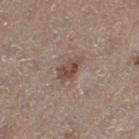{"biopsy_status": "not biopsied; imaged during a skin examination", "patient": {"sex": "female", "age_approx": 50}, "lighting": "white-light", "automated_metrics": {"area_mm2_approx": 5.5, "cielab_L": 47, "cielab_a": 16, "cielab_b": 24, "vs_skin_darker_L": 10.0, "vs_skin_contrast_norm": 8.0, "border_irregularity_0_10": 2.5, "color_variation_0_10": 3.5, "peripheral_color_asymmetry": 1.0, "lesion_detection_confidence_0_100": 100}, "lesion_size": {"long_diameter_mm_approx": 3.5}, "site": "leg", "image": {"source": "total-body photography crop", "field_of_view_mm": 15}}